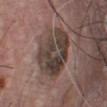Imaged during a routine full-body skin examination; the lesion was not biopsied and no histopathology is available. The patient is a male roughly 75 years of age. The lesion is located on the chest. A 15 mm crop from a total-body photograph taken for skin-cancer surveillance. Automated image analysis of the tile measured a footprint of about 24 mm², an eccentricity of roughly 0.65, and a shape-asymmetry score of about 0.2 (0 = symmetric). The analysis additionally found a lesion color around L≈40 a*≈13 b*≈20 in CIELAB and a lesion–skin lightness drop of about 11. It also reported a color-variation rating of about 7/10 and radial color variation of about 2.5. The recorded lesion diameter is about 6.5 mm.